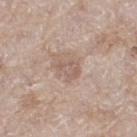Recorded during total-body skin imaging; not selected for excision or biopsy. A female patient aged 73–77. The lesion is located on the left thigh. A 15 mm close-up extracted from a 3D total-body photography capture. Longest diameter approximately 2.5 mm. Imaged with white-light lighting. The lesion-visualizer software estimated a lesion area of about 5 mm², an eccentricity of roughly 0.6, and two-axis asymmetry of about 0.4. The software also gave an average lesion color of about L≈58 a*≈16 b*≈24 (CIELAB), a lesion–skin lightness drop of about 8, and a normalized border contrast of about 5. And it measured a classifier nevus-likeness of about 0/100.